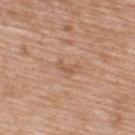The lesion was tiled from a total-body skin photograph and was not biopsied.
From the upper back.
The total-body-photography lesion software estimated a footprint of about 3 mm², an outline eccentricity of about 0.9 (0 = round, 1 = elongated), and two-axis asymmetry of about 0.4. It also reported a mean CIELAB color near L≈56 a*≈21 b*≈31, roughly 7 lightness units darker than nearby skin, and a normalized border contrast of about 5. The analysis additionally found a border-irregularity rating of about 4/10, a color-variation rating of about 0/10, and peripheral color asymmetry of about 0.
A roughly 15 mm field-of-view crop from a total-body skin photograph.
The patient is a male about 50 years old.
The tile uses white-light illumination.